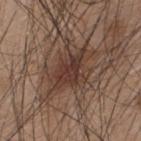notes: no biopsy performed (imaged during a skin exam)
subject: male, aged approximately 45
lighting: white-light
lesion size: about 5 mm
imaging modality: ~15 mm crop, total-body skin-cancer survey
anatomic site: the upper back